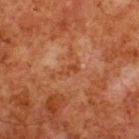Background:
A region of skin cropped from a whole-body photographic capture, roughly 15 mm wide. Imaged with cross-polarized lighting. A male subject aged 78 to 82. Located on the upper back. Approximately 2.5 mm at its widest.